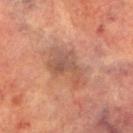follow-up = imaged on a skin check; not biopsied | lighting = cross-polarized | subject = male, aged 73–77 | site = the left lower leg | lesion diameter = ≈5.5 mm | automated lesion analysis = an automated nevus-likeness rating near 0 out of 100 and lesion-presence confidence of about 100/100 | acquisition = total-body-photography crop, ~15 mm field of view.